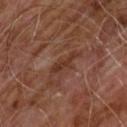On the chest. A 15 mm close-up tile from a total-body photography series done for melanoma screening. The lesion-visualizer software estimated a lesion area of about 3.5 mm², an outline eccentricity of about 0.95 (0 = round, 1 = elongated), and a shape-asymmetry score of about 0.45 (0 = symmetric). And it measured a lesion-to-skin contrast of about 7.5 (normalized; higher = more distinct). The analysis additionally found border irregularity of about 6 on a 0–10 scale and a peripheral color-asymmetry measure near 0. And it measured a nevus-likeness score of about 0/100. The patient is a male roughly 60 years of age. Approximately 3.5 mm at its widest. The tile uses cross-polarized illumination.